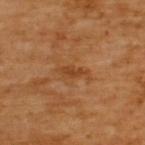Assessment:
The lesion was photographed on a routine skin check and not biopsied; there is no pathology result.
Clinical summary:
The lesion-visualizer software estimated a border-irregularity rating of about 4/10, a color-variation rating of about 0.5/10, and peripheral color asymmetry of about 0. Imaged with cross-polarized lighting. A male subject, aged 63 to 67. Cropped from a whole-body photographic skin survey; the tile spans about 15 mm. Located on the upper back. About 3.5 mm across.